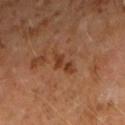Acquisition and patient details: The lesion is located on the arm. The lesion-visualizer software estimated a border-irregularity rating of about 5.5/10, a within-lesion color-variation index near 0.5/10, and radial color variation of about 0. And it measured a classifier nevus-likeness of about 0/100 and lesion-presence confidence of about 100/100. A male patient about 60 years old. Measured at roughly 4 mm in maximum diameter. This image is a 15 mm lesion crop taken from a total-body photograph.